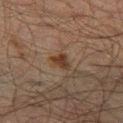image source: ~15 mm tile from a whole-body skin photo | body site: the left thigh | patient: male, aged approximately 50.